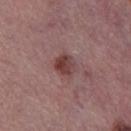biopsy_status: not biopsied; imaged during a skin examination
site: left thigh
lighting: white-light
patient:
  sex: female
  age_approx: 55
lesion_size:
  long_diameter_mm_approx: 2.5
image:
  source: total-body photography crop
  field_of_view_mm: 15